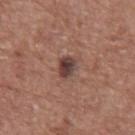Captured during whole-body skin photography for melanoma surveillance; the lesion was not biopsied.
On the abdomen.
Approximately 2.5 mm at its widest.
A lesion tile, about 15 mm wide, cut from a 3D total-body photograph.
A male subject, aged 73–77.